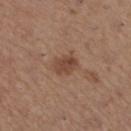Captured during whole-body skin photography for melanoma surveillance; the lesion was not biopsied. The patient is a female aged approximately 45. On the left lower leg. A 15 mm close-up tile from a total-body photography series done for melanoma screening. The recorded lesion diameter is about 3 mm.